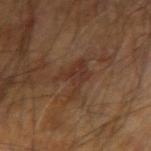Recorded during total-body skin imaging; not selected for excision or biopsy. This image is a 15 mm lesion crop taken from a total-body photograph. The lesion is located on the right arm. A male patient in their 60s. Measured at roughly 4 mm in maximum diameter.